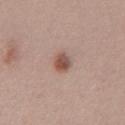The lesion was photographed on a routine skin check and not biopsied; there is no pathology result.
The lesion is on the abdomen.
A close-up tile cropped from a whole-body skin photograph, about 15 mm across.
A male patient, in their 40s.
Captured under white-light illumination.
The lesion's longest dimension is about 2.5 mm.